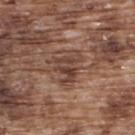{"biopsy_status": "not biopsied; imaged during a skin examination", "image": {"source": "total-body photography crop", "field_of_view_mm": 15}, "patient": {"sex": "male", "age_approx": 75}, "site": "back"}